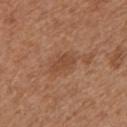Notes:
* workup — no biopsy performed (imaged during a skin exam)
* imaging modality — total-body-photography crop, ~15 mm field of view
* anatomic site — the left upper arm
* patient — female, aged around 40